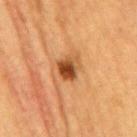follow-up: catalogued during a skin exam; not biopsied
patient: female, in their mid- to late 50s
automated metrics: a lesion color around L≈39 a*≈22 b*≈34 in CIELAB, about 14 CIELAB-L* units darker than the surrounding skin, and a lesion-to-skin contrast of about 11 (normalized; higher = more distinct); a lesion-detection confidence of about 100/100
lesion diameter: about 2.5 mm
image source: total-body-photography crop, ~15 mm field of view
location: the mid back
tile lighting: cross-polarized